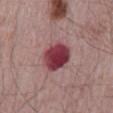The lesion was photographed on a routine skin check and not biopsied; there is no pathology result. The subject is a male aged approximately 65. The lesion is located on the back. A roughly 15 mm field-of-view crop from a total-body skin photograph.